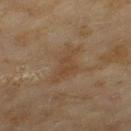  biopsy_status: not biopsied; imaged during a skin examination
  automated_metrics:
    eccentricity: 0.85
    shape_asymmetry: 0.45
    cielab_L: 40
    cielab_a: 14
    cielab_b: 28
    vs_skin_darker_L: 5.0
    vs_skin_contrast_norm: 5.0
    border_irregularity_0_10: 5.0
    color_variation_0_10: 2.5
    lesion_detection_confidence_0_100: 100
  lighting: cross-polarized
  lesion_size:
    long_diameter_mm_approx: 5.5
  patient:
    sex: female
    age_approx: 60
  site: left thigh
  image:
    source: total-body photography crop
    field_of_view_mm: 15